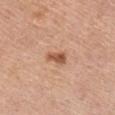The lesion was tiled from a total-body skin photograph and was not biopsied.
The total-body-photography lesion software estimated a footprint of about 3.5 mm², an outline eccentricity of about 0.8 (0 = round, 1 = elongated), and two-axis asymmetry of about 0.35. The analysis additionally found a lesion color around L≈55 a*≈24 b*≈34 in CIELAB and a lesion-to-skin contrast of about 9 (normalized; higher = more distinct).
The tile uses white-light illumination.
The patient is a female in their mid- to late 50s.
Located on the chest.
A 15 mm close-up extracted from a 3D total-body photography capture.